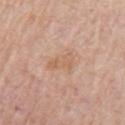The lesion was tiled from a total-body skin photograph and was not biopsied.
Automated image analysis of the tile measured a border-irregularity index near 4/10, internal color variation of about 3 on a 0–10 scale, and radial color variation of about 1. It also reported a nevus-likeness score of about 0/100.
A male subject aged 73 to 77.
Measured at roughly 3.5 mm in maximum diameter.
A roughly 15 mm field-of-view crop from a total-body skin photograph.
The lesion is located on the left upper arm.